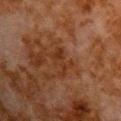A roughly 15 mm field-of-view crop from a total-body skin photograph.
A male subject, aged approximately 80.
The lesion is located on the chest.